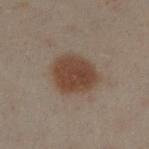Captured during whole-body skin photography for melanoma surveillance; the lesion was not biopsied.
From the left leg.
Cropped from a whole-body photographic skin survey; the tile spans about 15 mm.
The lesion-visualizer software estimated an outline eccentricity of about 0.5 (0 = round, 1 = elongated) and a shape-asymmetry score of about 0.15 (0 = symmetric). And it measured about 9 CIELAB-L* units darker than the surrounding skin. And it measured a nevus-likeness score of about 100/100 and a lesion-detection confidence of about 100/100.
The patient is a male in their 50s.
The tile uses cross-polarized illumination.